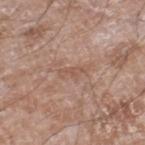Clinical impression: Imaged during a routine full-body skin examination; the lesion was not biopsied and no histopathology is available. Clinical summary: About 3 mm across. A male subject, aged approximately 60. Automated image analysis of the tile measured a lesion area of about 3 mm² and a shape eccentricity near 0.9. The software also gave about 6 CIELAB-L* units darker than the surrounding skin and a normalized border contrast of about 5. And it measured a detector confidence of about 100 out of 100 that the crop contains a lesion. A lesion tile, about 15 mm wide, cut from a 3D total-body photograph. Located on the right lower leg. The tile uses white-light illumination.